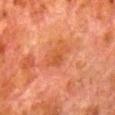notes = total-body-photography surveillance lesion; no biopsy | lesion diameter = about 3 mm | patient = male, aged 78–82 | acquisition = ~15 mm crop, total-body skin-cancer survey | body site = the right lower leg.